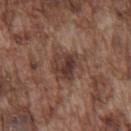The lesion was tiled from a total-body skin photograph and was not biopsied. The subject is a male approximately 75 years of age. Located on the chest. Imaged with white-light lighting. Approximately 4 mm at its widest. A close-up tile cropped from a whole-body skin photograph, about 15 mm across.